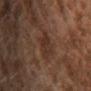Recorded during total-body skin imaging; not selected for excision or biopsy. A female patient, aged around 60. A 15 mm close-up extracted from a 3D total-body photography capture. The lesion's longest dimension is about 3 mm. The lesion is on the head or neck. Automated tile analysis of the lesion measured a shape eccentricity near 0.5 and two-axis asymmetry of about 0.2. It also reported a lesion color around L≈26 a*≈16 b*≈22 in CIELAB, roughly 5 lightness units darker than nearby skin, and a lesion-to-skin contrast of about 5.5 (normalized; higher = more distinct). It also reported a border-irregularity rating of about 2.5/10 and radial color variation of about 1. The software also gave an automated nevus-likeness rating near 0 out of 100 and lesion-presence confidence of about 100/100.